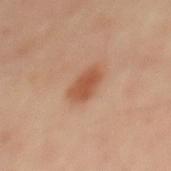Assessment:
The lesion was tiled from a total-body skin photograph and was not biopsied.
Background:
Located on the mid back. The tile uses cross-polarized illumination. An algorithmic analysis of the crop reported a mean CIELAB color near L≈52 a*≈24 b*≈33, a lesion–skin lightness drop of about 10, and a normalized border contrast of about 8. And it measured a border-irregularity rating of about 2/10, a within-lesion color-variation index near 2/10, and a peripheral color-asymmetry measure near 0.5. A male patient approximately 50 years of age. Cropped from a total-body skin-imaging series; the visible field is about 15 mm.